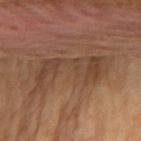{"biopsy_status": "not biopsied; imaged during a skin examination", "automated_metrics": {"eccentricity": 0.95, "shape_asymmetry": 0.5, "cielab_L": 43, "cielab_a": 18, "cielab_b": 29, "vs_skin_darker_L": 8.0, "vs_skin_contrast_norm": 6.5, "border_irregularity_0_10": 8.5, "color_variation_0_10": 3.5, "peripheral_color_asymmetry": 1.0}, "lesion_size": {"long_diameter_mm_approx": 9.5}, "image": {"source": "total-body photography crop", "field_of_view_mm": 15}, "lighting": "cross-polarized", "patient": {"sex": "female", "age_approx": 55}, "site": "left upper arm"}